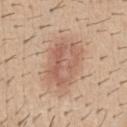| feature | finding |
|---|---|
| workup | catalogued during a skin exam; not biopsied |
| body site | the abdomen |
| subject | male, about 40 years old |
| imaging modality | ~15 mm tile from a whole-body skin photo |